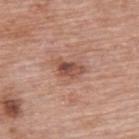The lesion was tiled from a total-body skin photograph and was not biopsied.
Measured at roughly 3.5 mm in maximum diameter.
Cropped from a whole-body photographic skin survey; the tile spans about 15 mm.
From the upper back.
Automated tile analysis of the lesion measured a shape eccentricity near 0.8 and a shape-asymmetry score of about 0.35 (0 = symmetric). And it measured a lesion color around L≈51 a*≈23 b*≈28 in CIELAB and about 11 CIELAB-L* units darker than the surrounding skin.
Imaged with white-light lighting.
A female subject aged around 60.